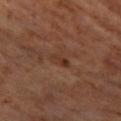lighting=cross-polarized illumination
acquisition=~15 mm crop, total-body skin-cancer survey
subject=male, roughly 60 years of age
location=the left thigh